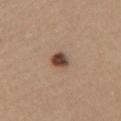Impression:
Imaged during a routine full-body skin examination; the lesion was not biopsied and no histopathology is available.
Clinical summary:
Cropped from a total-body skin-imaging series; the visible field is about 15 mm. The tile uses white-light illumination. A female subject, aged around 60. On the chest.